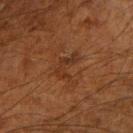biopsy status: no biopsy performed (imaged during a skin exam)
size: about 3.5 mm
subject: male, about 60 years old
location: the left forearm
imaging modality: ~15 mm tile from a whole-body skin photo
tile lighting: cross-polarized illumination